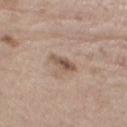| field | value |
|---|---|
| workup | catalogued during a skin exam; not biopsied |
| illumination | white-light |
| location | the left thigh |
| acquisition | ~15 mm tile from a whole-body skin photo |
| subject | male, approximately 70 years of age |